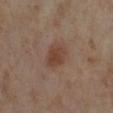Case summary:
* follow-up · total-body-photography surveillance lesion; no biopsy
* image-analysis metrics · a mean CIELAB color near L≈42 a*≈19 b*≈27, about 8 CIELAB-L* units darker than the surrounding skin, and a lesion-to-skin contrast of about 7.5 (normalized; higher = more distinct); a border-irregularity index near 1.5/10; an automated nevus-likeness rating near 90 out of 100 and a lesion-detection confidence of about 100/100
* lesion diameter · ~3.5 mm (longest diameter)
* patient · female, about 55 years old
* body site · the right lower leg
* imaging modality · ~15 mm crop, total-body skin-cancer survey